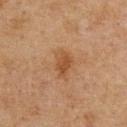Recorded during total-body skin imaging; not selected for excision or biopsy. Approximately 3.5 mm at its widest. Cropped from a whole-body photographic skin survey; the tile spans about 15 mm. On the abdomen. This is a cross-polarized tile. An algorithmic analysis of the crop reported an area of roughly 5.5 mm², a shape eccentricity near 0.75, and a symmetry-axis asymmetry near 0.25. The software also gave a lesion-detection confidence of about 100/100. The patient is a male aged around 75.